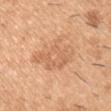notes: total-body-photography surveillance lesion; no biopsy
anatomic site: the arm
imaging modality: total-body-photography crop, ~15 mm field of view
patient: male, aged 38 to 42